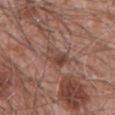Imaged during a routine full-body skin examination; the lesion was not biopsied and no histopathology is available. The lesion's longest dimension is about 4.5 mm. From the lower back. This is a white-light tile. A roughly 15 mm field-of-view crop from a total-body skin photograph. The lesion-visualizer software estimated roughly 8 lightness units darker than nearby skin and a lesion-to-skin contrast of about 6 (normalized; higher = more distinct). The software also gave a border-irregularity index near 5/10, a color-variation rating of about 6.5/10, and peripheral color asymmetry of about 2. A male patient, aged around 60.